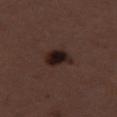Assessment:
Imaged during a routine full-body skin examination; the lesion was not biopsied and no histopathology is available.
Image and clinical context:
A region of skin cropped from a whole-body photographic capture, roughly 15 mm wide. From the right thigh. A female patient, about 50 years old.